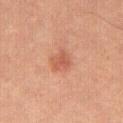The lesion was tiled from a total-body skin photograph and was not biopsied.
On the abdomen.
A female patient, in their mid- to late 50s.
Imaged with cross-polarized lighting.
The lesion's longest dimension is about 2.5 mm.
Cropped from a total-body skin-imaging series; the visible field is about 15 mm.